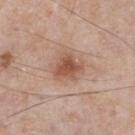The lesion was tiled from a total-body skin photograph and was not biopsied. A male subject, aged approximately 50. Measured at roughly 3.5 mm in maximum diameter. A 15 mm close-up extracted from a 3D total-body photography capture. The lesion-visualizer software estimated a border-irregularity index near 3.5/10, a within-lesion color-variation index near 3.5/10, and peripheral color asymmetry of about 1. Captured under white-light illumination. The lesion is located on the front of the torso.